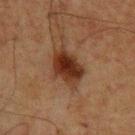Impression:
No biopsy was performed on this lesion — it was imaged during a full skin examination and was not determined to be concerning.
Background:
A lesion tile, about 15 mm wide, cut from a 3D total-body photograph. The patient is a male aged 58–62. The recorded lesion diameter is about 4 mm. On the back.